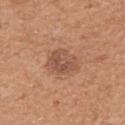Impression:
The lesion was tiled from a total-body skin photograph and was not biopsied.
Background:
The subject is a female in their mid-50s. A 15 mm close-up extracted from a 3D total-body photography capture. Located on the right upper arm.